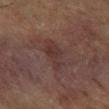Q: Is there a histopathology result?
A: catalogued during a skin exam; not biopsied
Q: What is the anatomic site?
A: the left thigh
Q: What kind of image is this?
A: ~15 mm crop, total-body skin-cancer survey
Q: What is the lesion's diameter?
A: ~4.5 mm (longest diameter)
Q: What are the patient's age and sex?
A: male, aged 63–67